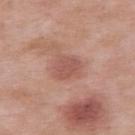<record>
<biopsy_status>not biopsied; imaged during a skin examination</biopsy_status>
<lesion_size>
  <long_diameter_mm_approx>3.0</long_diameter_mm_approx>
</lesion_size>
<site>left upper arm</site>
<image>
  <source>total-body photography crop</source>
  <field_of_view_mm>15</field_of_view_mm>
</image>
<patient>
  <sex>male</sex>
  <age_approx>55</age_approx>
</patient>
<automated_metrics>
  <cielab_L>54</cielab_L>
  <cielab_a>24</cielab_a>
  <cielab_b>26</cielab_b>
  <vs_skin_darker_L>8.0</vs_skin_darker_L>
  <vs_skin_contrast_norm>6.0</vs_skin_contrast_norm>
</automated_metrics>
<lighting>white-light</lighting>
</record>